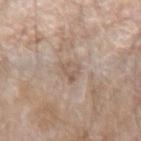The lesion was tiled from a total-body skin photograph and was not biopsied. The total-body-photography lesion software estimated a mean CIELAB color near L≈57 a*≈14 b*≈26, a lesion–skin lightness drop of about 7, and a normalized border contrast of about 5.5. The software also gave a classifier nevus-likeness of about 0/100 and a detector confidence of about 95 out of 100 that the crop contains a lesion. Approximately 3 mm at its widest. The subject is a male roughly 80 years of age. A 15 mm close-up extracted from a 3D total-body photography capture. This is a white-light tile. Located on the right forearm.